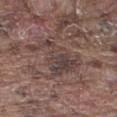biopsy status=total-body-photography surveillance lesion; no biopsy
image-analysis metrics=an area of roughly 15 mm² and a shape eccentricity near 0.8; an average lesion color of about L≈40 a*≈15 b*≈18 (CIELAB), a lesion–skin lightness drop of about 8, and a normalized lesion–skin contrast near 7
acquisition=15 mm crop, total-body photography
body site=the leg
illumination=white-light
patient=male, aged 73–77